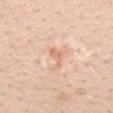The lesion was photographed on a routine skin check and not biopsied; there is no pathology result. Imaged with white-light lighting. Measured at roughly 3 mm in maximum diameter. On the mid back. Cropped from a whole-body photographic skin survey; the tile spans about 15 mm. The total-body-photography lesion software estimated a mean CIELAB color near L≈70 a*≈22 b*≈33, about 9 CIELAB-L* units darker than the surrounding skin, and a lesion-to-skin contrast of about 5.5 (normalized; higher = more distinct). And it measured a border-irregularity index near 6.5/10 and peripheral color asymmetry of about 0. A female subject, roughly 40 years of age.